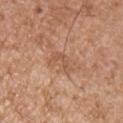Recorded during total-body skin imaging; not selected for excision or biopsy. A male subject, aged around 75. Captured under white-light illumination. The lesion's longest dimension is about 3 mm. Automated tile analysis of the lesion measured a nevus-likeness score of about 0/100. The lesion is located on the right upper arm. This image is a 15 mm lesion crop taken from a total-body photograph.